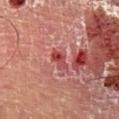Part of a total-body skin-imaging series; this lesion was reviewed on a skin check and was not flagged for biopsy. Captured under cross-polarized illumination. A male patient aged 53 to 57. The lesion is located on the right lower leg. Cropped from a total-body skin-imaging series; the visible field is about 15 mm. Measured at roughly 3 mm in maximum diameter. The lesion-visualizer software estimated a lesion area of about 3.5 mm², a shape eccentricity near 0.85, and a shape-asymmetry score of about 0.4 (0 = symmetric). It also reported a mean CIELAB color near L≈41 a*≈31 b*≈24, a lesion–skin lightness drop of about 9, and a lesion-to-skin contrast of about 7.5 (normalized; higher = more distinct).